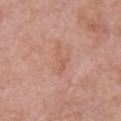– follow-up — imaged on a skin check; not biopsied
– anatomic site — the chest
– acquisition — total-body-photography crop, ~15 mm field of view
– subject — female, aged 58 to 62
– automated lesion analysis — a lesion area of about 3.5 mm², an outline eccentricity of about 0.95 (0 = round, 1 = elongated), and a shape-asymmetry score of about 0.45 (0 = symmetric); a mean CIELAB color near L≈58 a*≈23 b*≈30 and about 6 CIELAB-L* units darker than the surrounding skin; a border-irregularity index near 5.5/10 and radial color variation of about 0
– illumination — white-light
– size — ≈3.5 mm